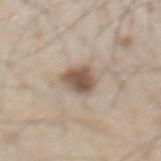{
  "patient": {
    "sex": "male",
    "age_approx": 60
  },
  "lesion_size": {
    "long_diameter_mm_approx": 3.5
  },
  "site": "mid back",
  "image": {
    "source": "total-body photography crop",
    "field_of_view_mm": 15
  }
}